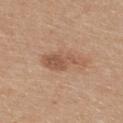Background: This image is a 15 mm lesion crop taken from a total-body photograph. On the upper back. The lesion-visualizer software estimated a lesion color around L≈54 a*≈20 b*≈31 in CIELAB, roughly 10 lightness units darker than nearby skin, and a lesion-to-skin contrast of about 6.5 (normalized; higher = more distinct). The software also gave a border-irregularity index near 4.5/10, a within-lesion color-variation index near 3/10, and radial color variation of about 1. The subject is a female roughly 30 years of age. About 5.5 mm across.